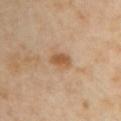workup: total-body-photography surveillance lesion; no biopsy | image: ~15 mm tile from a whole-body skin photo | patient: female, aged around 40 | site: the chest.